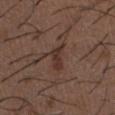<tbp_lesion>
<biopsy_status>not biopsied; imaged during a skin examination</biopsy_status>
<patient>
  <sex>male</sex>
  <age_approx>50</age_approx>
</patient>
<image>
  <source>total-body photography crop</source>
  <field_of_view_mm>15</field_of_view_mm>
</image>
<site>chest</site>
</tbp_lesion>